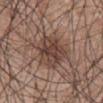Impression:
Part of a total-body skin-imaging series; this lesion was reviewed on a skin check and was not flagged for biopsy.
Image and clinical context:
The patient is a male aged 58 to 62. The lesion is on the abdomen. A 15 mm close-up tile from a total-body photography series done for melanoma screening.